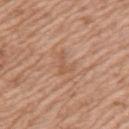The lesion was tiled from a total-body skin photograph and was not biopsied. The tile uses white-light illumination. About 3 mm across. A roughly 15 mm field-of-view crop from a total-body skin photograph. Located on the arm. A female patient, aged 73–77.